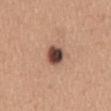biopsy_status: not biopsied; imaged during a skin examination
patient:
  sex: female
  age_approx: 30
image:
  source: total-body photography crop
  field_of_view_mm: 15
automated_metrics:
  cielab_L: 47
  cielab_a: 20
  cielab_b: 25
  vs_skin_contrast_norm: 13.0
lesion_size:
  long_diameter_mm_approx: 3.0
site: mid back
lighting: white-light